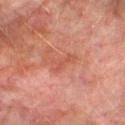Assessment: The lesion was tiled from a total-body skin photograph and was not biopsied. Context: The subject is a male in their mid-70s. Located on the right lower leg. A 15 mm close-up extracted from a 3D total-body photography capture.